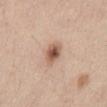Captured during whole-body skin photography for melanoma surveillance; the lesion was not biopsied.